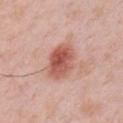This lesion was catalogued during total-body skin photography and was not selected for biopsy. Cropped from a whole-body photographic skin survey; the tile spans about 15 mm. The subject is a male in their mid-30s. The lesion is located on the chest.